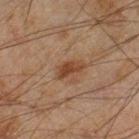Part of a total-body skin-imaging series; this lesion was reviewed on a skin check and was not flagged for biopsy.
Cropped from a total-body skin-imaging series; the visible field is about 15 mm.
This is a cross-polarized tile.
A male subject roughly 45 years of age.
About 3 mm across.
Located on the right lower leg.